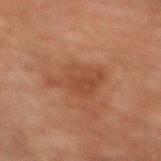workup=no biopsy performed (imaged during a skin exam); size=≈5.5 mm; lighting=cross-polarized; location=the left forearm; imaging modality=15 mm crop, total-body photography; patient=male, aged 73 to 77.